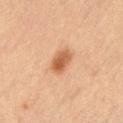Captured during whole-body skin photography for melanoma surveillance; the lesion was not biopsied.